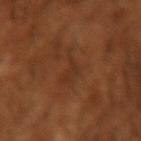Assessment:
The lesion was photographed on a routine skin check and not biopsied; there is no pathology result.
Acquisition and patient details:
The lesion-visualizer software estimated a normalized border contrast of about 5. The analysis additionally found border irregularity of about 8 on a 0–10 scale. A male patient, in their mid- to late 60s. This image is a 15 mm lesion crop taken from a total-body photograph. From the arm.